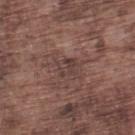Impression:
No biopsy was performed on this lesion — it was imaged during a full skin examination and was not determined to be concerning.
Context:
A male patient, in their mid-70s. The tile uses white-light illumination. On the left lower leg. Measured at roughly 2.5 mm in maximum diameter. The total-body-photography lesion software estimated a mean CIELAB color near L≈39 a*≈17 b*≈20, about 6 CIELAB-L* units darker than the surrounding skin, and a normalized border contrast of about 6. It also reported border irregularity of about 3 on a 0–10 scale, a color-variation rating of about 4/10, and peripheral color asymmetry of about 1.5. Cropped from a whole-body photographic skin survey; the tile spans about 15 mm.